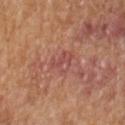This lesion was catalogued during total-body skin photography and was not selected for biopsy. A 15 mm crop from a total-body photograph taken for skin-cancer surveillance. On the left forearm. The subject is a female aged approximately 70.